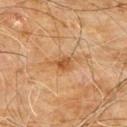Imaged during a routine full-body skin examination; the lesion was not biopsied and no histopathology is available.
An algorithmic analysis of the crop reported an area of roughly 4 mm², an outline eccentricity of about 0.75 (0 = round, 1 = elongated), and a shape-asymmetry score of about 0.45 (0 = symmetric). The analysis additionally found a border-irregularity index near 4.5/10, internal color variation of about 2 on a 0–10 scale, and a peripheral color-asymmetry measure near 0.5.
Imaged with cross-polarized lighting.
The subject is a male aged around 60.
Located on the chest.
Longest diameter approximately 3 mm.
A lesion tile, about 15 mm wide, cut from a 3D total-body photograph.